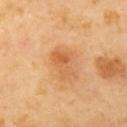Notes:
• workup: no biopsy performed (imaged during a skin exam)
• subject: female, aged 38–42
• lesion size: about 4 mm
• tile lighting: cross-polarized
• site: the left upper arm
• image source: ~15 mm crop, total-body skin-cancer survey
• automated metrics: a border-irregularity index near 4/10, a color-variation rating of about 6/10, and radial color variation of about 2; an automated nevus-likeness rating near 10 out of 100